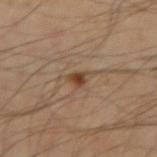biopsy status=no biopsy performed (imaged during a skin exam) | patient=male, in their mid-60s | body site=the right upper arm | illumination=cross-polarized illumination | image=~15 mm crop, total-body skin-cancer survey.